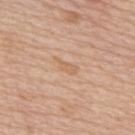- notes — no biopsy performed (imaged during a skin exam)
- subject — male, roughly 75 years of age
- diameter — ~2.5 mm (longest diameter)
- site — the upper back
- image source — 15 mm crop, total-body photography